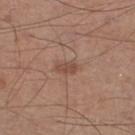tile lighting — white-light illumination
imaging modality — total-body-photography crop, ~15 mm field of view
subject — male, aged 58–62
body site — the right lower leg